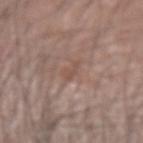Q: Was this lesion biopsied?
A: imaged on a skin check; not biopsied
Q: What are the patient's age and sex?
A: male, aged 43 to 47
Q: Automated lesion metrics?
A: an average lesion color of about L≈50 a*≈18 b*≈24 (CIELAB), roughly 6 lightness units darker than nearby skin, and a normalized lesion–skin contrast near 4.5
Q: What is the imaging modality?
A: total-body-photography crop, ~15 mm field of view
Q: What lighting was used for the tile?
A: white-light
Q: Lesion location?
A: the left forearm
Q: How large is the lesion?
A: about 2.5 mm